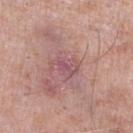Clinical summary:
The total-body-photography lesion software estimated a lesion area of about 6 mm², an eccentricity of roughly 0.75, and a symmetry-axis asymmetry near 0.4. It also reported internal color variation of about 3.5 on a 0–10 scale. And it measured a nevus-likeness score of about 0/100 and a detector confidence of about 90 out of 100 that the crop contains a lesion. The subject is a male in their mid- to late 70s. The lesion is located on the right lower leg. Captured under white-light illumination. Cropped from a total-body skin-imaging series; the visible field is about 15 mm. Approximately 3.5 mm at its widest.